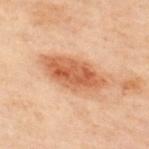follow-up — total-body-photography surveillance lesion; no biopsy
lesion diameter — ≈7.5 mm
subject — male, about 50 years old
TBP lesion metrics — about 11 CIELAB-L* units darker than the surrounding skin and a lesion-to-skin contrast of about 8.5 (normalized; higher = more distinct); an automated nevus-likeness rating near 95 out of 100 and lesion-presence confidence of about 100/100
lighting — cross-polarized illumination
image — 15 mm crop, total-body photography
location — the upper back